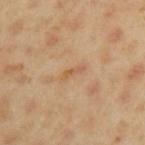A male patient roughly 45 years of age. Cropped from a total-body skin-imaging series; the visible field is about 15 mm. The lesion is on the left upper arm.